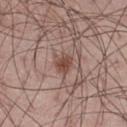Clinical impression:
The lesion was tiled from a total-body skin photograph and was not biopsied.
Clinical summary:
The lesion is on the right thigh. Automated tile analysis of the lesion measured a lesion color around L≈47 a*≈20 b*≈24 in CIELAB, about 10 CIELAB-L* units darker than the surrounding skin, and a normalized border contrast of about 8. The software also gave an automated nevus-likeness rating near 85 out of 100 and a lesion-detection confidence of about 100/100. A 15 mm crop from a total-body photograph taken for skin-cancer surveillance. Longest diameter approximately 2.5 mm. A male patient approximately 60 years of age. The tile uses white-light illumination.